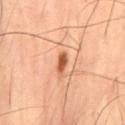biopsy status = no biopsy performed (imaged during a skin exam)
imaging modality = ~15 mm crop, total-body skin-cancer survey
location = the lower back
image-analysis metrics = an average lesion color of about L≈55 a*≈28 b*≈38 (CIELAB), about 15 CIELAB-L* units darker than the surrounding skin, and a normalized border contrast of about 10; border irregularity of about 3.5 on a 0–10 scale, internal color variation of about 0.5 on a 0–10 scale, and radial color variation of about 0
patient = male, aged around 55
lighting = cross-polarized illumination